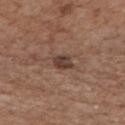{"biopsy_status": "not biopsied; imaged during a skin examination", "patient": {"sex": "male", "age_approx": 65}, "site": "mid back", "image": {"source": "total-body photography crop", "field_of_view_mm": 15}}